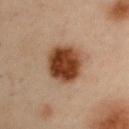Clinical impression: No biopsy was performed on this lesion — it was imaged during a full skin examination and was not determined to be concerning. Context: Located on the right upper arm. A male patient, approximately 55 years of age. A lesion tile, about 15 mm wide, cut from a 3D total-body photograph. The total-body-photography lesion software estimated an average lesion color of about L≈33 a*≈19 b*≈28 (CIELAB), roughly 16 lightness units darker than nearby skin, and a lesion-to-skin contrast of about 14 (normalized; higher = more distinct). It also reported a lesion-detection confidence of about 100/100. Imaged with cross-polarized lighting. The lesion's longest dimension is about 4.5 mm.